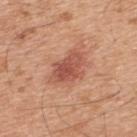Impression: Recorded during total-body skin imaging; not selected for excision or biopsy.